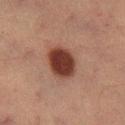notes: total-body-photography surveillance lesion; no biopsy | anatomic site: the right lower leg | lesion diameter: ~4.5 mm (longest diameter) | subject: female, aged 53–57 | image: ~15 mm tile from a whole-body skin photo | illumination: cross-polarized illumination.